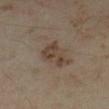Q: Was a biopsy performed?
A: no biopsy performed (imaged during a skin exam)
Q: What is the imaging modality?
A: ~15 mm tile from a whole-body skin photo
Q: What lighting was used for the tile?
A: cross-polarized illumination
Q: Who is the patient?
A: female, approximately 35 years of age
Q: Lesion size?
A: ≈4.5 mm
Q: Lesion location?
A: the leg